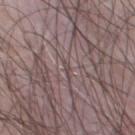The tile uses white-light illumination. From the chest. A 15 mm close-up extracted from a 3D total-body photography capture. The lesion's longest dimension is about 1 mm. The total-body-photography lesion software estimated a mean CIELAB color near L≈46 a*≈14 b*≈16 and roughly 7 lightness units darker than nearby skin. The analysis additionally found a nevus-likeness score of about 0/100 and a detector confidence of about 0 out of 100 that the crop contains a lesion. The patient is a male about 65 years old.